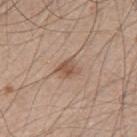The patient is a male aged 48–52. From the upper back. This image is a 15 mm lesion crop taken from a total-body photograph. Approximately 2.5 mm at its widest.